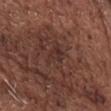follow-up: catalogued during a skin exam; not biopsied | size: ~3.5 mm (longest diameter) | illumination: white-light illumination | anatomic site: the chest | subject: male, aged approximately 75 | image-analysis metrics: a footprint of about 6 mm², an eccentricity of roughly 0.8, and a symmetry-axis asymmetry near 0.4 | image: ~15 mm tile from a whole-body skin photo.